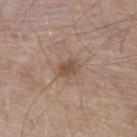– lighting · white-light illumination
– automated metrics · an area of roughly 4 mm², a shape eccentricity near 0.65, and a symmetry-axis asymmetry near 0.2; a border-irregularity rating of about 2/10 and peripheral color asymmetry of about 1; a detector confidence of about 100 out of 100 that the crop contains a lesion
– imaging modality · ~15 mm crop, total-body skin-cancer survey
– patient · male, aged 53 to 57
– diameter · about 3 mm
– site · the upper back